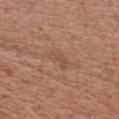No biopsy was performed on this lesion — it was imaged during a full skin examination and was not determined to be concerning. The lesion is located on the chest. A female subject aged 58–62. Automated tile analysis of the lesion measured a classifier nevus-likeness of about 0/100 and a detector confidence of about 100 out of 100 that the crop contains a lesion. This image is a 15 mm lesion crop taken from a total-body photograph. The tile uses white-light illumination. Approximately 3 mm at its widest.